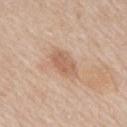Q: Was this lesion biopsied?
A: no biopsy performed (imaged during a skin exam)
Q: What is the anatomic site?
A: the upper back
Q: What is the imaging modality?
A: total-body-photography crop, ~15 mm field of view
Q: Who is the patient?
A: male, aged approximately 60